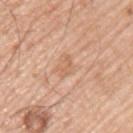{
  "biopsy_status": "not biopsied; imaged during a skin examination",
  "lesion_size": {
    "long_diameter_mm_approx": 2.5
  },
  "image": {
    "source": "total-body photography crop",
    "field_of_view_mm": 15
  },
  "site": "left upper arm",
  "lighting": "white-light",
  "patient": {
    "sex": "male",
    "age_approx": 55
  }
}